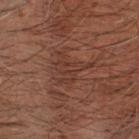<record>
  <lesion_size>
    <long_diameter_mm_approx>4.5</long_diameter_mm_approx>
  </lesion_size>
  <image>
    <source>total-body photography crop</source>
    <field_of_view_mm>15</field_of_view_mm>
  </image>
  <patient>
    <sex>male</sex>
    <age_approx>65</age_approx>
  </patient>
  <automated_metrics>
    <cielab_L>35</cielab_L>
    <cielab_a>20</cielab_a>
    <cielab_b>25</cielab_b>
    <vs_skin_darker_L>5.0</vs_skin_darker_L>
    <vs_skin_contrast_norm>5.0</vs_skin_contrast_norm>
  </automated_metrics>
  <lighting>cross-polarized</lighting>
</record>